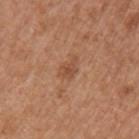* notes: catalogued during a skin exam; not biopsied
* patient: male, aged 63–67
* tile lighting: white-light illumination
* automated lesion analysis: an area of roughly 3.5 mm² and an eccentricity of roughly 0.75; a lesion color around L≈50 a*≈22 b*≈33 in CIELAB and a lesion–skin lightness drop of about 8
* image: ~15 mm tile from a whole-body skin photo
* size: ≈2.5 mm
* body site: the left upper arm